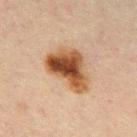workup=total-body-photography surveillance lesion; no biopsy | lighting=cross-polarized | subject=male, in their 70s | site=the mid back | imaging modality=total-body-photography crop, ~15 mm field of view.